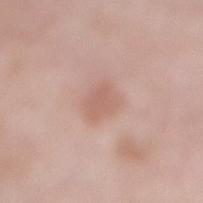Clinical impression: This lesion was catalogued during total-body skin photography and was not selected for biopsy.